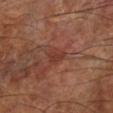– workup — imaged on a skin check; not biopsied
– diameter — ≈2.5 mm
– image source — total-body-photography crop, ~15 mm field of view
– tile lighting — cross-polarized
– subject — male, approximately 60 years of age
– site — the left lower leg